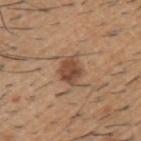Q: Was this lesion biopsied?
A: total-body-photography surveillance lesion; no biopsy
Q: What is the imaging modality?
A: 15 mm crop, total-body photography
Q: Where on the body is the lesion?
A: the left upper arm
Q: Patient demographics?
A: male, in their 60s